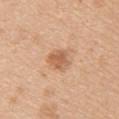- biopsy status — imaged on a skin check; not biopsied
- patient — female, roughly 40 years of age
- image — 15 mm crop, total-body photography
- image-analysis metrics — a lesion area of about 5.5 mm² and a shape-asymmetry score of about 0.2 (0 = symmetric); a lesion color around L≈60 a*≈22 b*≈35 in CIELAB, roughly 11 lightness units darker than nearby skin, and a normalized border contrast of about 7; a border-irregularity rating of about 2/10
- anatomic site — the upper back
- tile lighting — white-light illumination